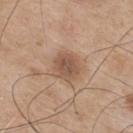The lesion is located on the mid back.
The recorded lesion diameter is about 3.5 mm.
The subject is a male aged 73–77.
A region of skin cropped from a whole-body photographic capture, roughly 15 mm wide.